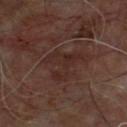biopsy status: no biopsy performed (imaged during a skin exam)
location: the upper back
lighting: cross-polarized illumination
TBP lesion metrics: a lesion area of about 11 mm² and an outline eccentricity of about 0.65 (0 = round, 1 = elongated); a mean CIELAB color near L≈25 a*≈17 b*≈20, about 5 CIELAB-L* units darker than the surrounding skin, and a normalized lesion–skin contrast near 6; a border-irregularity rating of about 9/10 and peripheral color asymmetry of about 1; an automated nevus-likeness rating near 0 out of 100
lesion size: ≈5 mm
subject: male, about 60 years old
image: ~15 mm tile from a whole-body skin photo